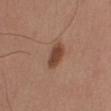Assessment: Recorded during total-body skin imaging; not selected for excision or biopsy. Acquisition and patient details: The lesion's longest dimension is about 3.5 mm. On the arm. A lesion tile, about 15 mm wide, cut from a 3D total-body photograph. A male patient approximately 55 years of age.